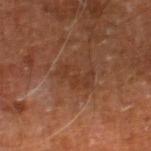Q: What are the patient's age and sex?
A: male, roughly 60 years of age
Q: How was the tile lit?
A: cross-polarized illumination
Q: What kind of image is this?
A: ~15 mm tile from a whole-body skin photo
Q: Where on the body is the lesion?
A: the right leg
Q: How large is the lesion?
A: about 4 mm